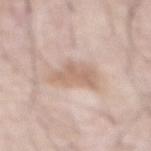{"biopsy_status": "not biopsied; imaged during a skin examination", "patient": {"sex": "male", "age_approx": 60}, "lesion_size": {"long_diameter_mm_approx": 4.0}, "automated_metrics": {"cielab_L": 64, "cielab_a": 17, "cielab_b": 28, "vs_skin_darker_L": 9.0, "border_irregularity_0_10": 5.0, "color_variation_0_10": 1.5, "peripheral_color_asymmetry": 0.5, "nevus_likeness_0_100": 0}, "image": {"source": "total-body photography crop", "field_of_view_mm": 15}, "lighting": "white-light", "site": "front of the torso"}